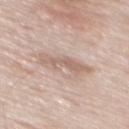Impression:
This lesion was catalogued during total-body skin photography and was not selected for biopsy.
Background:
The tile uses white-light illumination. A male subject aged 53–57. Measured at roughly 5 mm in maximum diameter. Automated tile analysis of the lesion measured about 9 CIELAB-L* units darker than the surrounding skin and a normalized lesion–skin contrast near 6. It also reported a border-irregularity index near 5.5/10 and internal color variation of about 2.5 on a 0–10 scale. The analysis additionally found an automated nevus-likeness rating near 15 out of 100. This image is a 15 mm lesion crop taken from a total-body photograph. From the back.